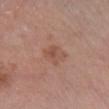Notes:
– notes · no biopsy performed (imaged during a skin exam)
– subject · male, about 65 years old
– acquisition · 15 mm crop, total-body photography
– body site · the leg
– automated metrics · a lesion color around L≈52 a*≈21 b*≈28 in CIELAB, a lesion–skin lightness drop of about 7, and a normalized lesion–skin contrast near 5.5; border irregularity of about 2.5 on a 0–10 scale, internal color variation of about 4 on a 0–10 scale, and radial color variation of about 1.5; an automated nevus-likeness rating near 0 out of 100 and a detector confidence of about 100 out of 100 that the crop contains a lesion
– lesion size · ~3 mm (longest diameter)